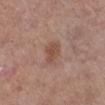Assessment: Recorded during total-body skin imaging; not selected for excision or biopsy. Acquisition and patient details: Longest diameter approximately 2.5 mm. The lesion is on the right lower leg. This image is a 15 mm lesion crop taken from a total-body photograph. The subject is a female approximately 60 years of age.